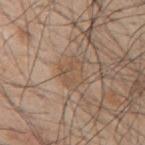Part of a total-body skin-imaging series; this lesion was reviewed on a skin check and was not flagged for biopsy.
Cropped from a total-body skin-imaging series; the visible field is about 15 mm.
The lesion is on the right upper arm.
The subject is a male in their 50s.